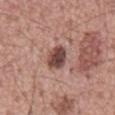Captured during whole-body skin photography for melanoma surveillance; the lesion was not biopsied. A male patient aged 53–57. The tile uses white-light illumination. On the mid back. About 3 mm across. A 15 mm close-up tile from a total-body photography series done for melanoma screening.